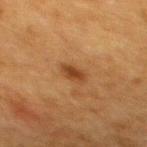<lesion>
  <biopsy_status>not biopsied; imaged during a skin examination</biopsy_status>
  <image>
    <source>total-body photography crop</source>
    <field_of_view_mm>15</field_of_view_mm>
  </image>
  <lighting>cross-polarized</lighting>
  <site>back</site>
  <lesion_size>
    <long_diameter_mm_approx>2.5</long_diameter_mm_approx>
  </lesion_size>
  <patient>
    <sex>female</sex>
    <age_approx>55</age_approx>
  </patient>
</lesion>